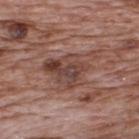biopsy status: no biopsy performed (imaged during a skin exam) | patient: male, roughly 70 years of age | location: the upper back | size: ≈6 mm | acquisition: 15 mm crop, total-body photography | lighting: white-light illumination | image-analysis metrics: an automated nevus-likeness rating near 10 out of 100 and a detector confidence of about 95 out of 100 that the crop contains a lesion.